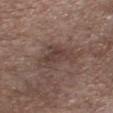| feature | finding |
|---|---|
| follow-up | catalogued during a skin exam; not biopsied |
| tile lighting | white-light |
| patient | male, in their 80s |
| image | total-body-photography crop, ~15 mm field of view |
| anatomic site | the chest |
| lesion size | ~5 mm (longest diameter) |